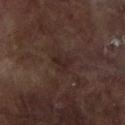Imaged during a routine full-body skin examination; the lesion was not biopsied and no histopathology is available.
A female subject aged approximately 80.
A 15 mm close-up tile from a total-body photography series done for melanoma screening.
This is a cross-polarized tile.
On the left arm.
An algorithmic analysis of the crop reported an area of roughly 3.5 mm² and two-axis asymmetry of about 0.5. The software also gave an average lesion color of about L≈21 a*≈14 b*≈15 (CIELAB), roughly 5 lightness units darker than nearby skin, and a normalized border contrast of about 6.5. It also reported a border-irregularity index near 4.5/10 and a peripheral color-asymmetry measure near 0.5. And it measured an automated nevus-likeness rating near 0 out of 100 and a detector confidence of about 100 out of 100 that the crop contains a lesion.